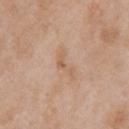The lesion was photographed on a routine skin check and not biopsied; there is no pathology result. The total-body-photography lesion software estimated an outline eccentricity of about 0.9 (0 = round, 1 = elongated). And it measured a nevus-likeness score of about 0/100 and lesion-presence confidence of about 100/100. Captured under white-light illumination. Located on the chest. A male subject aged 48 to 52. This image is a 15 mm lesion crop taken from a total-body photograph. About 3 mm across.